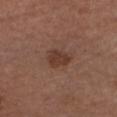notes: catalogued during a skin exam; not biopsied | acquisition: ~15 mm tile from a whole-body skin photo | body site: the head or neck | image-analysis metrics: a lesion area of about 5.5 mm², an outline eccentricity of about 0.6 (0 = round, 1 = elongated), and a shape-asymmetry score of about 0.25 (0 = symmetric); an average lesion color of about L≈37 a*≈20 b*≈26 (CIELAB) and a lesion–skin lightness drop of about 8 | subject: male, aged 73 to 77.